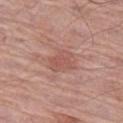Clinical impression:
Part of a total-body skin-imaging series; this lesion was reviewed on a skin check and was not flagged for biopsy.
Clinical summary:
A male subject aged 73 to 77. This image is a 15 mm lesion crop taken from a total-body photograph. About 3 mm across. The lesion is located on the left thigh.